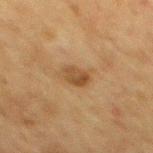Clinical impression:
Recorded during total-body skin imaging; not selected for excision or biopsy.
Acquisition and patient details:
The subject is a male in their mid- to late 70s. The tile uses cross-polarized illumination. From the mid back. A 15 mm close-up tile from a total-body photography series done for melanoma screening. The recorded lesion diameter is about 2.5 mm.